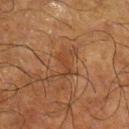Impression: No biopsy was performed on this lesion — it was imaged during a full skin examination and was not determined to be concerning. Acquisition and patient details: On the leg. A male subject, aged 63–67. An algorithmic analysis of the crop reported an eccentricity of roughly 0.9 and a symmetry-axis asymmetry near 0.6. It also reported about 5 CIELAB-L* units darker than the surrounding skin and a lesion-to-skin contrast of about 5 (normalized; higher = more distinct). The analysis additionally found a border-irregularity index near 7.5/10, internal color variation of about 2 on a 0–10 scale, and peripheral color asymmetry of about 0.5. The tile uses cross-polarized illumination. This image is a 15 mm lesion crop taken from a total-body photograph.